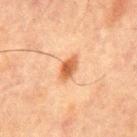Imaged during a routine full-body skin examination; the lesion was not biopsied and no histopathology is available. The lesion is located on the chest. A roughly 15 mm field-of-view crop from a total-body skin photograph. Captured under cross-polarized illumination. About 3.5 mm across. The lesion-visualizer software estimated an area of roughly 4.5 mm² and a symmetry-axis asymmetry near 0.2. A male patient roughly 65 years of age.